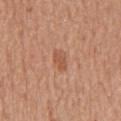Case summary:
– workup · imaged on a skin check; not biopsied
– lighting · white-light illumination
– patient · male, in their mid-60s
– diameter · ~2.5 mm (longest diameter)
– TBP lesion metrics · an average lesion color of about L≈54 a*≈24 b*≈33 (CIELAB), about 8 CIELAB-L* units darker than the surrounding skin, and a normalized border contrast of about 6
– location · the chest
– acquisition · 15 mm crop, total-body photography